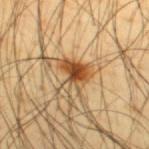No biopsy was performed on this lesion — it was imaged during a full skin examination and was not determined to be concerning. A roughly 15 mm field-of-view crop from a total-body skin photograph. Longest diameter approximately 4 mm. From the upper back. A male patient in their mid-40s.